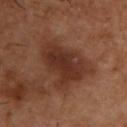Findings:
- notes · catalogued during a skin exam; not biopsied
- tile lighting · cross-polarized illumination
- anatomic site · the upper back
- size · ≈8 mm
- TBP lesion metrics · a border-irregularity rating of about 5/10, a color-variation rating of about 4/10, and a peripheral color-asymmetry measure near 1
- subject · male, aged around 55
- acquisition · ~15 mm tile from a whole-body skin photo